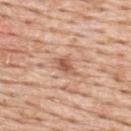Q: Was a biopsy performed?
A: catalogued during a skin exam; not biopsied
Q: How was this image acquired?
A: 15 mm crop, total-body photography
Q: What are the patient's age and sex?
A: male, aged approximately 60
Q: What is the lesion's diameter?
A: about 3 mm
Q: How was the tile lit?
A: white-light illumination
Q: Automated lesion metrics?
A: a lesion area of about 4 mm², a shape eccentricity near 0.8, and a symmetry-axis asymmetry near 0.25; a nevus-likeness score of about 5/100 and a detector confidence of about 100 out of 100 that the crop contains a lesion
Q: Lesion location?
A: the upper back